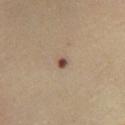Imaged during a routine full-body skin examination; the lesion was not biopsied and no histopathology is available. The lesion is located on the right lower leg. A male subject aged 38–42. A close-up tile cropped from a whole-body skin photograph, about 15 mm across.